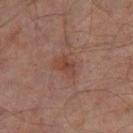Impression:
The lesion was tiled from a total-body skin photograph and was not biopsied.
Image and clinical context:
The recorded lesion diameter is about 3 mm. A male subject approximately 65 years of age. A close-up tile cropped from a whole-body skin photograph, about 15 mm across. The tile uses cross-polarized illumination. Located on the right thigh. The lesion-visualizer software estimated a mean CIELAB color near L≈41 a*≈20 b*≈26. The analysis additionally found a border-irregularity rating of about 3/10, a within-lesion color-variation index near 3.5/10, and a peripheral color-asymmetry measure near 1. It also reported a detector confidence of about 100 out of 100 that the crop contains a lesion.